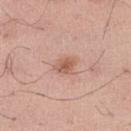follow-up: imaged on a skin check; not biopsied
patient: male, approximately 40 years of age
site: the left thigh
image source: ~15 mm tile from a whole-body skin photo